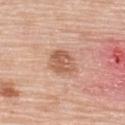biopsy status — imaged on a skin check; not biopsied.